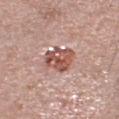Q: Is there a histopathology result?
A: no biopsy performed (imaged during a skin exam)
Q: What is the imaging modality?
A: total-body-photography crop, ~15 mm field of view
Q: Lesion location?
A: the head or neck
Q: What is the lesion's diameter?
A: ~3.5 mm (longest diameter)
Q: What are the patient's age and sex?
A: female, aged 58–62
Q: How was the tile lit?
A: white-light